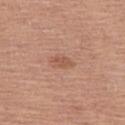| feature | finding |
|---|---|
| follow-up | imaged on a skin check; not biopsied |
| image source | 15 mm crop, total-body photography |
| anatomic site | the left thigh |
| subject | female, approximately 50 years of age |
| tile lighting | white-light |